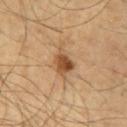Imaged during a routine full-body skin examination; the lesion was not biopsied and no histopathology is available. The lesion is located on the mid back. This is a cross-polarized tile. A 15 mm close-up tile from a total-body photography series done for melanoma screening. A male subject, aged approximately 60.